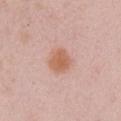notes: imaged on a skin check; not biopsied | anatomic site: the chest | acquisition: ~15 mm crop, total-body skin-cancer survey | diameter: ~3 mm (longest diameter) | lighting: white-light illumination | patient: female, aged 58 to 62.